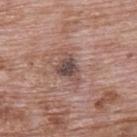follow-up — total-body-photography surveillance lesion; no biopsy | patient — male, approximately 70 years of age | diameter — ≈4 mm | site — the upper back | acquisition — 15 mm crop, total-body photography.